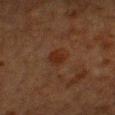The lesion was photographed on a routine skin check and not biopsied; there is no pathology result.
This is a cross-polarized tile.
From the head or neck.
A female subject, aged approximately 55.
Measured at roughly 3 mm in maximum diameter.
Cropped from a whole-body photographic skin survey; the tile spans about 15 mm.